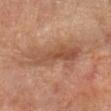workup: imaged on a skin check; not biopsied | patient: male, aged approximately 55 | body site: the left forearm | tile lighting: cross-polarized | diameter: about 7.5 mm | image: total-body-photography crop, ~15 mm field of view | image-analysis metrics: a shape eccentricity near 0.95 and a shape-asymmetry score of about 0.35 (0 = symmetric).